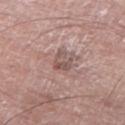Recorded during total-body skin imaging; not selected for excision or biopsy. This is a white-light tile. A male patient roughly 75 years of age. A close-up tile cropped from a whole-body skin photograph, about 15 mm across. The lesion is located on the left thigh. Approximately 2.5 mm at its widest.